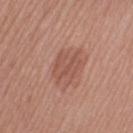Q: Where on the body is the lesion?
A: the right thigh
Q: Lesion size?
A: about 4.5 mm
Q: Automated lesion metrics?
A: a shape eccentricity near 0.65 and two-axis asymmetry of about 0.3; a mean CIELAB color near L≈53 a*≈23 b*≈27, a lesion–skin lightness drop of about 8, and a lesion-to-skin contrast of about 5.5 (normalized; higher = more distinct); a nevus-likeness score of about 10/100
Q: What kind of image is this?
A: total-body-photography crop, ~15 mm field of view
Q: What are the patient's age and sex?
A: female, roughly 55 years of age
Q: Illumination type?
A: white-light illumination